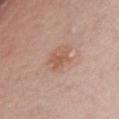This lesion was catalogued during total-body skin photography and was not selected for biopsy. A roughly 15 mm field-of-view crop from a total-body skin photograph. From the abdomen. A male patient, aged approximately 75. Captured under white-light illumination.